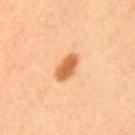Assessment:
Part of a total-body skin-imaging series; this lesion was reviewed on a skin check and was not flagged for biopsy.
Context:
A female subject, aged approximately 50. Cropped from a total-body skin-imaging series; the visible field is about 15 mm. Automated tile analysis of the lesion measured a shape eccentricity near 0.85 and two-axis asymmetry of about 0.2. It also reported a border-irregularity index near 2/10 and a peripheral color-asymmetry measure near 0.5.